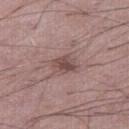Clinical summary:
A male patient, aged 48 to 52. This is a white-light tile. Located on the right thigh. About 3 mm across. A 15 mm close-up extracted from a 3D total-body photography capture.